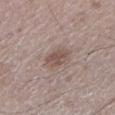  patient:
    sex: male
    age_approx: 50
  automated_metrics:
    cielab_L: 51
    cielab_a: 15
    cielab_b: 22
    vs_skin_darker_L: 9.0
    border_irregularity_0_10: 1.5
    color_variation_0_10: 3.0
    peripheral_color_asymmetry: 1.0
    nevus_likeness_0_100: 50
    lesion_detection_confidence_0_100: 100
  image:
    source: total-body photography crop
    field_of_view_mm: 15
  site: left lower leg
  lighting: white-light